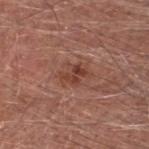image source: total-body-photography crop, ~15 mm field of view | site: the head or neck | subject: male, in their mid-50s.